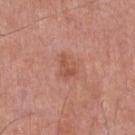Measured at roughly 3.5 mm in maximum diameter.
This image is a 15 mm lesion crop taken from a total-body photograph.
Automated tile analysis of the lesion measured a footprint of about 6.5 mm², an outline eccentricity of about 0.65 (0 = round, 1 = elongated), and a symmetry-axis asymmetry near 0.4. The software also gave a mean CIELAB color near L≈54 a*≈25 b*≈30, a lesion–skin lightness drop of about 7, and a lesion-to-skin contrast of about 5.5 (normalized; higher = more distinct). It also reported a color-variation rating of about 3/10. And it measured an automated nevus-likeness rating near 10 out of 100.
Imaged with white-light lighting.
A male patient aged around 55.
On the chest.